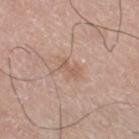The lesion was tiled from a total-body skin photograph and was not biopsied.
Automated tile analysis of the lesion measured a lesion area of about 5 mm² and two-axis asymmetry of about 0.25. And it measured a border-irregularity rating of about 3/10, a within-lesion color-variation index near 2/10, and a peripheral color-asymmetry measure near 0.5.
The lesion is on the right thigh.
Imaged with white-light lighting.
Measured at roughly 3 mm in maximum diameter.
A close-up tile cropped from a whole-body skin photograph, about 15 mm across.
The patient is a male aged approximately 75.